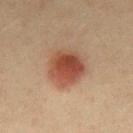Clinical impression:
Part of a total-body skin-imaging series; this lesion was reviewed on a skin check and was not flagged for biopsy.
Context:
Located on the mid back. Automated image analysis of the tile measured a mean CIELAB color near L≈44 a*≈21 b*≈28 and a lesion–skin lightness drop of about 12. It also reported a classifier nevus-likeness of about 100/100 and a detector confidence of about 100 out of 100 that the crop contains a lesion. This is a cross-polarized tile. A roughly 15 mm field-of-view crop from a total-body skin photograph. A male patient aged 38–42. The lesion's longest dimension is about 5 mm.